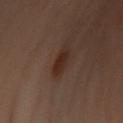{
  "biopsy_status": "not biopsied; imaged during a skin examination",
  "automated_metrics": {
    "area_mm2_approx": 4.5,
    "eccentricity": 0.75,
    "shape_asymmetry": 0.2,
    "cielab_L": 22,
    "cielab_a": 16,
    "cielab_b": 20,
    "vs_skin_darker_L": 7.0,
    "vs_skin_contrast_norm": 8.5,
    "border_irregularity_0_10": 2.5,
    "color_variation_0_10": 1.5,
    "peripheral_color_asymmetry": 0.5,
    "nevus_likeness_0_100": 85
  },
  "lesion_size": {
    "long_diameter_mm_approx": 3.0
  },
  "image": {
    "source": "total-body photography crop",
    "field_of_view_mm": 15
  },
  "patient": {
    "sex": "female",
    "age_approx": 60
  },
  "site": "back",
  "lighting": "cross-polarized"
}